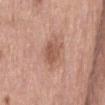Findings:
- notes: no biopsy performed (imaged during a skin exam)
- patient: male, about 70 years old
- imaging modality: total-body-photography crop, ~15 mm field of view
- lesion size: about 3.5 mm
- image-analysis metrics: a border-irregularity index near 2/10 and a within-lesion color-variation index near 3/10
- lighting: white-light illumination
- anatomic site: the abdomen